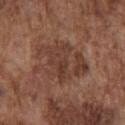Imaged during a routine full-body skin examination; the lesion was not biopsied and no histopathology is available.
A male subject aged 73–77.
This is a white-light tile.
The lesion is on the chest.
Cropped from a total-body skin-imaging series; the visible field is about 15 mm.